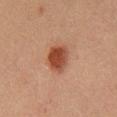Captured during whole-body skin photography for melanoma surveillance; the lesion was not biopsied. From the front of the torso. A 15 mm close-up extracted from a 3D total-body photography capture. Imaged with cross-polarized lighting. Approximately 3.5 mm at its widest. An algorithmic analysis of the crop reported an area of roughly 8 mm², an outline eccentricity of about 0.7 (0 = round, 1 = elongated), and a shape-asymmetry score of about 0.2 (0 = symmetric). It also reported about 11 CIELAB-L* units darker than the surrounding skin and a lesion-to-skin contrast of about 10 (normalized; higher = more distinct). The software also gave a color-variation rating of about 3/10 and peripheral color asymmetry of about 1. A male patient, aged 48 to 52.